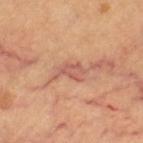{"biopsy_status": "not biopsied; imaged during a skin examination", "lighting": "cross-polarized", "patient": {"sex": "female", "age_approx": 60}, "site": "left thigh", "lesion_size": {"long_diameter_mm_approx": 4.0}, "image": {"source": "total-body photography crop", "field_of_view_mm": 15}}